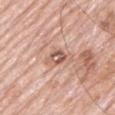workup = imaged on a skin check; not biopsied | patient = male, aged 78 to 82 | location = the mid back | illumination = white-light | imaging modality = ~15 mm tile from a whole-body skin photo.